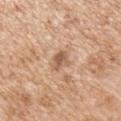The lesion was photographed on a routine skin check and not biopsied; there is no pathology result. A male patient roughly 65 years of age. A 15 mm close-up tile from a total-body photography series done for melanoma screening. Located on the right upper arm. Imaged with white-light lighting. Approximately 2.5 mm at its widest.